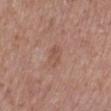notes: catalogued during a skin exam; not biopsied | subject: female, aged approximately 75 | imaging modality: ~15 mm crop, total-body skin-cancer survey | body site: the leg | TBP lesion metrics: an area of roughly 4 mm², an outline eccentricity of about 0.7 (0 = round, 1 = elongated), and a shape-asymmetry score of about 0.3 (0 = symmetric); an average lesion color of about L≈52 a*≈21 b*≈27 (CIELAB), a lesion–skin lightness drop of about 6, and a normalized lesion–skin contrast near 5; a border-irregularity index near 3/10, a within-lesion color-variation index near 2/10, and a peripheral color-asymmetry measure near 0.5; an automated nevus-likeness rating near 0 out of 100 and a detector confidence of about 100 out of 100 that the crop contains a lesion | lighting: white-light.